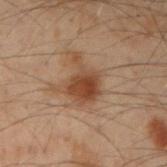<record>
<biopsy_status>not biopsied; imaged during a skin examination</biopsy_status>
<image>
  <source>total-body photography crop</source>
  <field_of_view_mm>15</field_of_view_mm>
</image>
<lesion_size>
  <long_diameter_mm_approx>4.5</long_diameter_mm_approx>
</lesion_size>
<site>left forearm</site>
<automated_metrics>
  <area_mm2_approx>10.0</area_mm2_approx>
  <eccentricity>0.7</eccentricity>
  <shape_asymmetry>0.45</shape_asymmetry>
  <cielab_L>36</cielab_L>
  <cielab_a>17</cielab_a>
  <cielab_b>27</cielab_b>
  <vs_skin_darker_L>9.0</vs_skin_darker_L>
  <vs_skin_contrast_norm>9.0</vs_skin_contrast_norm>
</automated_metrics>
<patient>
  <sex>male</sex>
  <age_approx>50</age_approx>
</patient>
<lighting>cross-polarized</lighting>
</record>